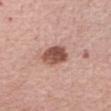Recorded during total-body skin imaging; not selected for excision or biopsy. Cropped from a whole-body photographic skin survey; the tile spans about 15 mm. The lesion is located on the right forearm. A female patient, in their mid- to late 60s. Longest diameter approximately 3 mm.